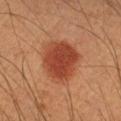Q: Was this lesion biopsied?
A: no biopsy performed (imaged during a skin exam)
Q: Who is the patient?
A: male, aged 53 to 57
Q: What kind of image is this?
A: ~15 mm crop, total-body skin-cancer survey
Q: Lesion location?
A: the arm
Q: What lighting was used for the tile?
A: cross-polarized illumination